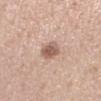Assessment:
Part of a total-body skin-imaging series; this lesion was reviewed on a skin check and was not flagged for biopsy.
Acquisition and patient details:
A 15 mm close-up extracted from a 3D total-body photography capture. From the left forearm. The total-body-photography lesion software estimated a lesion area of about 5.5 mm², an outline eccentricity of about 0.65 (0 = round, 1 = elongated), and a shape-asymmetry score of about 0.15 (0 = symmetric). The analysis additionally found roughly 13 lightness units darker than nearby skin and a normalized lesion–skin contrast near 8. The analysis additionally found a border-irregularity rating of about 1.5/10, a color-variation rating of about 4/10, and a peripheral color-asymmetry measure near 1.5. This is a white-light tile. The subject is a female aged 48–52.